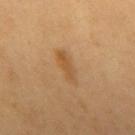Context: Cropped from a whole-body photographic skin survey; the tile spans about 15 mm. The patient is a male aged around 60. From the mid back.